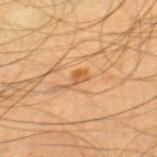Notes:
• workup — total-body-photography surveillance lesion; no biopsy
• body site — the right thigh
• patient — male, aged 33–37
• tile lighting — cross-polarized
• image — ~15 mm crop, total-body skin-cancer survey